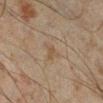Imaged during a routine full-body skin examination; the lesion was not biopsied and no histopathology is available.
Automated image analysis of the tile measured a footprint of about 4 mm², an outline eccentricity of about 0.65 (0 = round, 1 = elongated), and a symmetry-axis asymmetry near 0.45. The software also gave a within-lesion color-variation index near 2/10 and radial color variation of about 1.
This image is a 15 mm lesion crop taken from a total-body photograph.
The patient is a male about 45 years old.
From the right lower leg.
The tile uses cross-polarized illumination.
About 3 mm across.